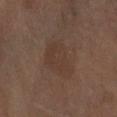The tile uses cross-polarized illumination. The subject is a female aged 68–72. A region of skin cropped from a whole-body photographic capture, roughly 15 mm wide. The recorded lesion diameter is about 5 mm. The lesion is on the arm.